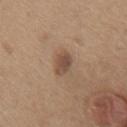<lesion>
<biopsy_status>not biopsied; imaged during a skin examination</biopsy_status>
<site>chest</site>
<patient>
  <sex>male</sex>
  <age_approx>65</age_approx>
</patient>
<automated_metrics>
  <nevus_likeness_0_100>50</nevus_likeness_0_100>
  <lesion_detection_confidence_0_100>100</lesion_detection_confidence_0_100>
</automated_metrics>
<lesion_size>
  <long_diameter_mm_approx>3.0</long_diameter_mm_approx>
</lesion_size>
<lighting>white-light</lighting>
<image>
  <source>total-body photography crop</source>
  <field_of_view_mm>15</field_of_view_mm>
</image>
</lesion>